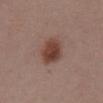workup: no biopsy performed (imaged during a skin exam)
subject: female, aged approximately 55
lesion diameter: ~4 mm (longest diameter)
acquisition: ~15 mm tile from a whole-body skin photo
anatomic site: the front of the torso